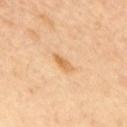From the upper back. A close-up tile cropped from a whole-body skin photograph, about 15 mm across. The subject is a male in their 60s. The tile uses cross-polarized illumination. Automated tile analysis of the lesion measured roughly 9 lightness units darker than nearby skin and a lesion-to-skin contrast of about 7.5 (normalized; higher = more distinct). The software also gave a nevus-likeness score of about 40/100 and a lesion-detection confidence of about 100/100.